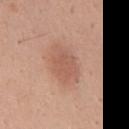Impression: Part of a total-body skin-imaging series; this lesion was reviewed on a skin check and was not flagged for biopsy. Background: Automated tile analysis of the lesion measured a lesion area of about 13 mm², a shape eccentricity near 0.75, and two-axis asymmetry of about 0.1. The software also gave a mean CIELAB color near L≈58 a*≈22 b*≈29, a lesion–skin lightness drop of about 8, and a normalized border contrast of about 5.5. And it measured a color-variation rating of about 2/10 and radial color variation of about 0.5. The software also gave a nevus-likeness score of about 85/100 and lesion-presence confidence of about 100/100. Approximately 5 mm at its widest. A roughly 15 mm field-of-view crop from a total-body skin photograph. The tile uses white-light illumination. The lesion is on the chest. The subject is a male roughly 40 years of age.